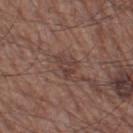Q: Was this lesion biopsied?
A: total-body-photography surveillance lesion; no biopsy
Q: What is the imaging modality?
A: 15 mm crop, total-body photography
Q: Lesion location?
A: the left thigh
Q: How large is the lesion?
A: ~3 mm (longest diameter)
Q: What did automated image analysis measure?
A: a lesion area of about 4.5 mm², a shape eccentricity near 0.75, and a shape-asymmetry score of about 0.45 (0 = symmetric); a nevus-likeness score of about 0/100 and a lesion-detection confidence of about 95/100
Q: Who is the patient?
A: male, in their 60s
Q: Illumination type?
A: white-light illumination